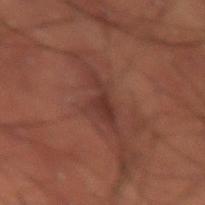Assessment:
This lesion was catalogued during total-body skin photography and was not selected for biopsy.
Background:
A 15 mm close-up tile from a total-body photography series done for melanoma screening. The lesion is located on the right thigh. Approximately 5 mm at its widest. The subject is a male aged approximately 50. Automated image analysis of the tile measured roughly 6 lightness units darker than nearby skin and a lesion-to-skin contrast of about 7 (normalized; higher = more distinct). The analysis additionally found a border-irregularity rating of about 7/10 and internal color variation of about 1.5 on a 0–10 scale. The analysis additionally found an automated nevus-likeness rating near 5 out of 100. This is a cross-polarized tile.